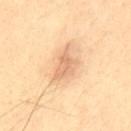Findings:
– notes · catalogued during a skin exam; not biopsied
– image source · 15 mm crop, total-body photography
– size · ~4 mm (longest diameter)
– location · the mid back
– tile lighting · cross-polarized illumination
– patient · male, in their 40s
– TBP lesion metrics · an area of roughly 6.5 mm², an outline eccentricity of about 0.8 (0 = round, 1 = elongated), and a symmetry-axis asymmetry near 0.45; a lesion color around L≈71 a*≈21 b*≈37 in CIELAB, roughly 10 lightness units darker than nearby skin, and a lesion-to-skin contrast of about 6 (normalized; higher = more distinct); a border-irregularity rating of about 4.5/10, internal color variation of about 2.5 on a 0–10 scale, and a peripheral color-asymmetry measure near 0.5; a nevus-likeness score of about 60/100